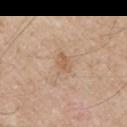Imaged during a routine full-body skin examination; the lesion was not biopsied and no histopathology is available. The recorded lesion diameter is about 2.5 mm. Cropped from a total-body skin-imaging series; the visible field is about 15 mm. From the left upper arm. Automated tile analysis of the lesion measured a lesion area of about 3 mm², an outline eccentricity of about 0.8 (0 = round, 1 = elongated), and two-axis asymmetry of about 0.3. And it measured a lesion color around L≈59 a*≈17 b*≈33 in CIELAB, a lesion–skin lightness drop of about 8, and a normalized border contrast of about 5.5. And it measured internal color variation of about 1 on a 0–10 scale. The analysis additionally found a nevus-likeness score of about 0/100 and a detector confidence of about 100 out of 100 that the crop contains a lesion. Captured under white-light illumination. A male patient approximately 60 years of age.